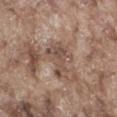Imaged during a routine full-body skin examination; the lesion was not biopsied and no histopathology is available.
From the right thigh.
A male patient, aged approximately 75.
A 15 mm crop from a total-body photograph taken for skin-cancer surveillance.
The lesion's longest dimension is about 4.5 mm.
Captured under white-light illumination.